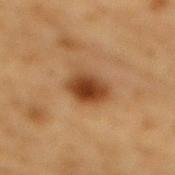The lesion was tiled from a total-body skin photograph and was not biopsied.
A lesion tile, about 15 mm wide, cut from a 3D total-body photograph.
An algorithmic analysis of the crop reported an automated nevus-likeness rating near 100 out of 100 and a lesion-detection confidence of about 100/100.
The tile uses cross-polarized illumination.
A male patient, aged 83 or older.
The lesion is located on the back.